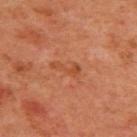Assessment: Captured during whole-body skin photography for melanoma surveillance; the lesion was not biopsied. Context: Imaged with cross-polarized lighting. Located on the chest. This image is a 15 mm lesion crop taken from a total-body photograph. Longest diameter approximately 3.5 mm. The patient is a male aged 58 to 62.